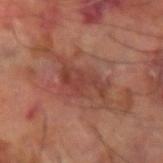Assessment: Recorded during total-body skin imaging; not selected for excision or biopsy. Acquisition and patient details: About 5.5 mm across. On the right arm. Cropped from a total-body skin-imaging series; the visible field is about 15 mm. The subject is a male aged around 60. This is a cross-polarized tile.